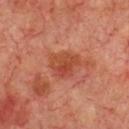notes: no biopsy performed (imaged during a skin exam) | automated metrics: a footprint of about 8.5 mm², a shape eccentricity near 0.55, and a shape-asymmetry score of about 0.3 (0 = symmetric); a border-irregularity rating of about 3/10, internal color variation of about 6 on a 0–10 scale, and a peripheral color-asymmetry measure near 2; a classifier nevus-likeness of about 15/100 and lesion-presence confidence of about 100/100 | image source: ~15 mm crop, total-body skin-cancer survey | tile lighting: cross-polarized illumination | patient: male, aged around 70 | body site: the chest.